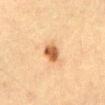follow-up: no biopsy performed (imaged during a skin exam); image: total-body-photography crop, ~15 mm field of view; patient: male, aged around 50; site: the abdomen.